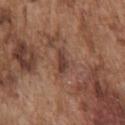Assessment:
Imaged during a routine full-body skin examination; the lesion was not biopsied and no histopathology is available.
Clinical summary:
A 15 mm crop from a total-body photograph taken for skin-cancer surveillance. A male patient, in their mid- to late 70s. From the chest. The tile uses white-light illumination.